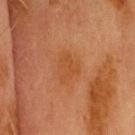Notes:
* workup — catalogued during a skin exam; not biopsied
* diameter — about 3.5 mm
* tile lighting — cross-polarized illumination
* anatomic site — the head or neck
* acquisition — ~15 mm tile from a whole-body skin photo
* patient — male, about 70 years old